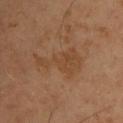The lesion-visualizer software estimated roughly 5 lightness units darker than nearby skin and a normalized border contrast of about 5. The analysis additionally found a classifier nevus-likeness of about 0/100 and a detector confidence of about 100 out of 100 that the crop contains a lesion.
A 15 mm close-up extracted from a 3D total-body photography capture.
Located on the upper back.
The patient is a male aged 48 to 52.